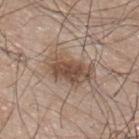On the upper back.
A region of skin cropped from a whole-body photographic capture, roughly 15 mm wide.
This is a white-light tile.
The subject is a male aged approximately 45.
The lesion's longest dimension is about 5.5 mm.
The total-body-photography lesion software estimated a lesion area of about 15 mm², an outline eccentricity of about 0.8 (0 = round, 1 = elongated), and two-axis asymmetry of about 0.25. The analysis additionally found a border-irregularity index near 3/10.